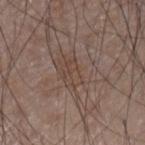This lesion was catalogued during total-body skin photography and was not selected for biopsy. Measured at roughly 3 mm in maximum diameter. This image is a 15 mm lesion crop taken from a total-body photograph. A male subject in their mid-70s. From the left forearm. Captured under white-light illumination.